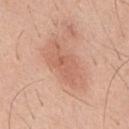| field | value |
|---|---|
| biopsy status | imaged on a skin check; not biopsied |
| patient | male, aged around 40 |
| size | about 5.5 mm |
| anatomic site | the mid back |
| illumination | white-light |
| imaging modality | ~15 mm crop, total-body skin-cancer survey |